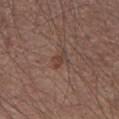workup=no biopsy performed (imaged during a skin exam) | diameter=~3 mm (longest diameter) | illumination=white-light illumination | subject=male, approximately 55 years of age | image-analysis metrics=a border-irregularity index near 3.5/10 and internal color variation of about 0.5 on a 0–10 scale; a nevus-likeness score of about 5/100 | image source=total-body-photography crop, ~15 mm field of view | site=the right upper arm.